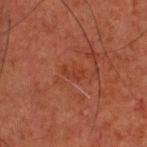workup: catalogued during a skin exam; not biopsied | image-analysis metrics: a footprint of about 3 mm², an outline eccentricity of about 0.9 (0 = round, 1 = elongated), and a shape-asymmetry score of about 0.4 (0 = symmetric) | anatomic site: the head or neck | image: ~15 mm crop, total-body skin-cancer survey | lesion size: ~2.5 mm (longest diameter) | subject: male, aged approximately 50 | illumination: cross-polarized illumination.